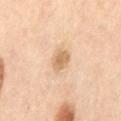No biopsy was performed on this lesion — it was imaged during a full skin examination and was not determined to be concerning. A close-up tile cropped from a whole-body skin photograph, about 15 mm across. On the back. Imaged with cross-polarized lighting. A male patient, approximately 65 years of age. The lesion's longest dimension is about 3 mm.